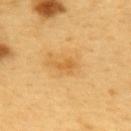notes=no biopsy performed (imaged during a skin exam)
image-analysis metrics=a mean CIELAB color near L≈61 a*≈21 b*≈48, a lesion–skin lightness drop of about 8, and a normalized border contrast of about 5.5; a nevus-likeness score of about 10/100 and lesion-presence confidence of about 100/100
anatomic site=the upper back
imaging modality=total-body-photography crop, ~15 mm field of view
diameter=about 3 mm
subject=male, aged approximately 60